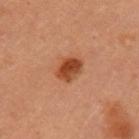Recorded during total-body skin imaging; not selected for excision or biopsy. A 15 mm crop from a total-body photograph taken for skin-cancer surveillance. A female patient in their mid-30s. Measured at roughly 3 mm in maximum diameter. The lesion is located on the arm. The tile uses cross-polarized illumination. The lesion-visualizer software estimated a lesion color around L≈45 a*≈28 b*≈37 in CIELAB, about 13 CIELAB-L* units darker than the surrounding skin, and a lesion-to-skin contrast of about 10.5 (normalized; higher = more distinct).